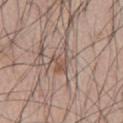Part of a total-body skin-imaging series; this lesion was reviewed on a skin check and was not flagged for biopsy.
A male subject, aged 68–72.
About 3.5 mm across.
Located on the abdomen.
An algorithmic analysis of the crop reported a classifier nevus-likeness of about 0/100 and a detector confidence of about 65 out of 100 that the crop contains a lesion.
Cropped from a whole-body photographic skin survey; the tile spans about 15 mm.